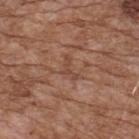| feature | finding |
|---|---|
| follow-up | imaged on a skin check; not biopsied |
| site | the upper back |
| patient | male, roughly 75 years of age |
| image source | ~15 mm crop, total-body skin-cancer survey |
| illumination | white-light |
| automated lesion analysis | an average lesion color of about L≈46 a*≈22 b*≈29 (CIELAB); a border-irregularity index near 8/10, a within-lesion color-variation index near 0/10, and radial color variation of about 0; an automated nevus-likeness rating near 0 out of 100 and lesion-presence confidence of about 90/100 |
| size | ≈3 mm |